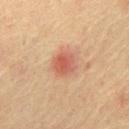notes: total-body-photography surveillance lesion; no biopsy | diameter: ~3.5 mm (longest diameter) | image: 15 mm crop, total-body photography | site: the front of the torso | patient: male, aged approximately 65 | illumination: cross-polarized illumination.